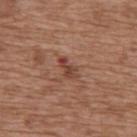Part of a total-body skin-imaging series; this lesion was reviewed on a skin check and was not flagged for biopsy.
Automated image analysis of the tile measured an area of roughly 3.5 mm² and a symmetry-axis asymmetry near 0.3. And it measured a mean CIELAB color near L≈43 a*≈24 b*≈27 and a normalized lesion–skin contrast near 7.5. And it measured a border-irregularity index near 3.5/10, internal color variation of about 2 on a 0–10 scale, and radial color variation of about 0.5.
The subject is a female in their mid-70s.
A region of skin cropped from a whole-body photographic capture, roughly 15 mm wide.
The lesion is on the upper back.